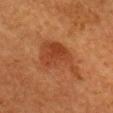  biopsy_status: not biopsied; imaged during a skin examination
  image:
    source: total-body photography crop
    field_of_view_mm: 15
  site: head or neck
  patient:
    sex: female
    age_approx: 50